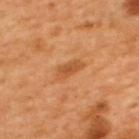Impression:
The lesion was photographed on a routine skin check and not biopsied; there is no pathology result.
Context:
Located on the upper back. An algorithmic analysis of the crop reported an area of roughly 4.5 mm², an eccentricity of roughly 0.9, and two-axis asymmetry of about 0.35. It also reported about 9 CIELAB-L* units darker than the surrounding skin and a normalized lesion–skin contrast near 6.5. And it measured a nevus-likeness score of about 55/100 and a lesion-detection confidence of about 100/100. Imaged with cross-polarized lighting. A 15 mm close-up tile from a total-body photography series done for melanoma screening. A male subject, aged approximately 50.